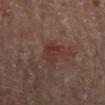The lesion was photographed on a routine skin check and not biopsied; there is no pathology result.
The lesion is on the mid back.
A 15 mm crop from a total-body photograph taken for skin-cancer surveillance.
Imaged with cross-polarized lighting.
The recorded lesion diameter is about 3.5 mm.
The lesion-visualizer software estimated a footprint of about 4.5 mm², an eccentricity of roughly 0.85, and a symmetry-axis asymmetry near 0.25. The analysis additionally found a lesion color around L≈32 a*≈20 b*≈22 in CIELAB. And it measured a border-irregularity index near 3/10, a within-lesion color-variation index near 3/10, and peripheral color asymmetry of about 1. And it measured a classifier nevus-likeness of about 0/100 and a lesion-detection confidence of about 100/100.
The subject is a male approximately 65 years of age.